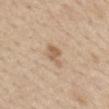Part of a total-body skin-imaging series; this lesion was reviewed on a skin check and was not flagged for biopsy. The patient is a female aged approximately 65. The lesion-visualizer software estimated a color-variation rating of about 2.5/10 and radial color variation of about 1. Imaged with white-light lighting. Longest diameter approximately 3 mm. The lesion is on the mid back. Cropped from a whole-body photographic skin survey; the tile spans about 15 mm.